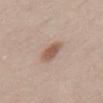Recorded during total-body skin imaging; not selected for excision or biopsy.
Imaged with white-light lighting.
Approximately 3.5 mm at its widest.
A female subject, aged 38–42.
The lesion is on the lower back.
A region of skin cropped from a whole-body photographic capture, roughly 15 mm wide.
The lesion-visualizer software estimated an automated nevus-likeness rating near 95 out of 100 and lesion-presence confidence of about 100/100.